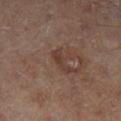Q: Is there a histopathology result?
A: catalogued during a skin exam; not biopsied
Q: Lesion location?
A: the leg
Q: What are the patient's age and sex?
A: male, aged around 65
Q: How was the tile lit?
A: cross-polarized
Q: Automated lesion metrics?
A: roughly 6 lightness units darker than nearby skin; a classifier nevus-likeness of about 0/100
Q: What kind of image is this?
A: ~15 mm tile from a whole-body skin photo